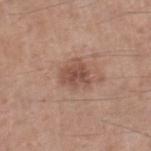Findings:
– follow-up: no biopsy performed (imaged during a skin exam)
– image source: ~15 mm crop, total-body skin-cancer survey
– diameter: ≈3.5 mm
– tile lighting: white-light
– subject: male, in their mid- to late 50s
– site: the right lower leg